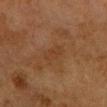<lesion>
  <biopsy_status>not biopsied; imaged during a skin examination</biopsy_status>
  <automated_metrics>
    <vs_skin_contrast_norm>5.0</vs_skin_contrast_norm>
  </automated_metrics>
  <image>
    <source>total-body photography crop</source>
    <field_of_view_mm>15</field_of_view_mm>
  </image>
  <patient>
    <sex>female</sex>
    <age_approx>55</age_approx>
  </patient>
  <lighting>cross-polarized</lighting>
  <lesion_size>
    <long_diameter_mm_approx>3.5</long_diameter_mm_approx>
  </lesion_size>
  <site>arm</site>
</lesion>